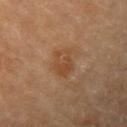notes — total-body-photography surveillance lesion; no biopsy | illumination — cross-polarized | acquisition — total-body-photography crop, ~15 mm field of view | site — the left upper arm | automated metrics — a lesion color around L≈44 a*≈19 b*≈33 in CIELAB, about 7 CIELAB-L* units darker than the surrounding skin, and a lesion-to-skin contrast of about 6 (normalized; higher = more distinct) | patient — male, aged 83 or over | diameter — ≈3.5 mm.